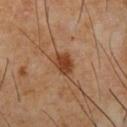{"biopsy_status": "not biopsied; imaged during a skin examination", "image": {"source": "total-body photography crop", "field_of_view_mm": 15}, "site": "chest", "patient": {"sex": "male", "age_approx": 65}}